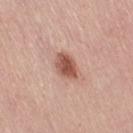Clinical impression: This lesion was catalogued during total-body skin photography and was not selected for biopsy. Image and clinical context: Imaged with white-light lighting. A roughly 15 mm field-of-view crop from a total-body skin photograph. A female patient, aged 48–52. Longest diameter approximately 3.5 mm. The lesion is located on the right thigh.